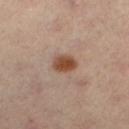biopsy_status: not biopsied; imaged during a skin examination
site: right leg
automated_metrics:
  cielab_L: 48
  cielab_a: 21
  cielab_b: 31
  vs_skin_contrast_norm: 10.5
image:
  source: total-body photography crop
  field_of_view_mm: 15
patient:
  sex: female
  age_approx: 40